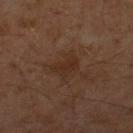Impression: Recorded during total-body skin imaging; not selected for excision or biopsy. Image and clinical context: A male subject, approximately 60 years of age. The lesion's longest dimension is about 3 mm. Automated tile analysis of the lesion measured a border-irregularity rating of about 4/10, a color-variation rating of about 1.5/10, and radial color variation of about 0.5. It also reported a classifier nevus-likeness of about 0/100. Located on the right thigh. This is a cross-polarized tile. A 15 mm crop from a total-body photograph taken for skin-cancer surveillance.